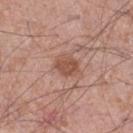{
  "biopsy_status": "not biopsied; imaged during a skin examination",
  "lesion_size": {
    "long_diameter_mm_approx": 3.0
  },
  "lighting": "white-light",
  "patient": {
    "sex": "male",
    "age_approx": 55
  },
  "image": {
    "source": "total-body photography crop",
    "field_of_view_mm": 15
  },
  "site": "leg"
}